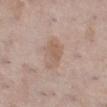<record>
<biopsy_status>not biopsied; imaged during a skin examination</biopsy_status>
<patient>
  <sex>female</sex>
  <age_approx>40</age_approx>
</patient>
<image>
  <source>total-body photography crop</source>
  <field_of_view_mm>15</field_of_view_mm>
</image>
<lesion_size>
  <long_diameter_mm_approx>4.0</long_diameter_mm_approx>
</lesion_size>
<site>right lower leg</site>
<lighting>white-light</lighting>
</record>